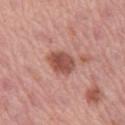Captured during whole-body skin photography for melanoma surveillance; the lesion was not biopsied. On the left thigh. Measured at roughly 3.5 mm in maximum diameter. A female subject, about 45 years old. A roughly 15 mm field-of-view crop from a total-body skin photograph. Imaged with white-light lighting. The lesion-visualizer software estimated a border-irregularity rating of about 1.5/10 and peripheral color asymmetry of about 1. The software also gave a classifier nevus-likeness of about 90/100 and a detector confidence of about 100 out of 100 that the crop contains a lesion.